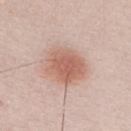{"biopsy_status": "not biopsied; imaged during a skin examination", "patient": {"sex": "male", "age_approx": 25}, "image": {"source": "total-body photography crop", "field_of_view_mm": 15}, "site": "chest"}